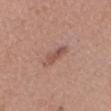Notes:
• workup: total-body-photography surveillance lesion; no biopsy
• patient: female, in their mid- to late 30s
• anatomic site: the head or neck
• lesion size: about 4 mm
• imaging modality: 15 mm crop, total-body photography
• image-analysis metrics: an area of roughly 5 mm², an eccentricity of roughly 0.9, and a shape-asymmetry score of about 0.4 (0 = symmetric); about 9 CIELAB-L* units darker than the surrounding skin; border irregularity of about 4.5 on a 0–10 scale, internal color variation of about 3 on a 0–10 scale, and peripheral color asymmetry of about 1; a classifier nevus-likeness of about 5/100 and lesion-presence confidence of about 100/100